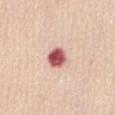Q: What is the imaging modality?
A: 15 mm crop, total-body photography
Q: What are the patient's age and sex?
A: female, about 55 years old
Q: What is the anatomic site?
A: the abdomen